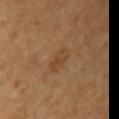Q: Was this lesion biopsied?
A: total-body-photography surveillance lesion; no biopsy
Q: Who is the patient?
A: female, aged around 50
Q: What kind of image is this?
A: total-body-photography crop, ~15 mm field of view
Q: What is the anatomic site?
A: the right upper arm